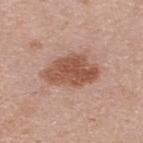<tbp_lesion>
<biopsy_status>not biopsied; imaged during a skin examination</biopsy_status>
<site>upper back</site>
<lighting>white-light</lighting>
<lesion_size>
  <long_diameter_mm_approx>6.0</long_diameter_mm_approx>
</lesion_size>
<patient>
  <sex>male</sex>
  <age_approx>45</age_approx>
</patient>
<image>
  <source>total-body photography crop</source>
  <field_of_view_mm>15</field_of_view_mm>
</image>
</tbp_lesion>